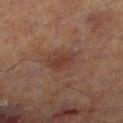This lesion was catalogued during total-body skin photography and was not selected for biopsy. The tile uses cross-polarized illumination. From the leg. Cropped from a whole-body photographic skin survey; the tile spans about 15 mm. A male subject approximately 70 years of age. Longest diameter approximately 3.5 mm. Automated tile analysis of the lesion measured an average lesion color of about L≈30 a*≈17 b*≈21 (CIELAB), about 6 CIELAB-L* units darker than the surrounding skin, and a normalized border contrast of about 6. And it measured border irregularity of about 3 on a 0–10 scale, internal color variation of about 2.5 on a 0–10 scale, and peripheral color asymmetry of about 1. And it measured a nevus-likeness score of about 40/100 and a detector confidence of about 100 out of 100 that the crop contains a lesion.